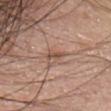Part of a total-body skin-imaging series; this lesion was reviewed on a skin check and was not flagged for biopsy. A female patient about 65 years old. A 15 mm close-up extracted from a 3D total-body photography capture. The lesion-visualizer software estimated border irregularity of about 3.5 on a 0–10 scale, internal color variation of about 0 on a 0–10 scale, and a peripheral color-asymmetry measure near 0. The software also gave a classifier nevus-likeness of about 0/100 and a detector confidence of about 55 out of 100 that the crop contains a lesion. Captured under white-light illumination. The lesion is on the head or neck. Measured at roughly 2.5 mm in maximum diameter.